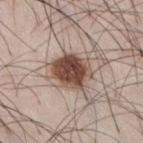Captured during whole-body skin photography for melanoma surveillance; the lesion was not biopsied. From the right thigh. Approximately 4 mm at its widest. Imaged with white-light lighting. Cropped from a total-body skin-imaging series; the visible field is about 15 mm. A male patient about 35 years old.